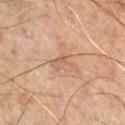Recorded during total-body skin imaging; not selected for excision or biopsy. The patient is a male aged 58–62. A close-up tile cropped from a whole-body skin photograph, about 15 mm across. The lesion is on the left leg.